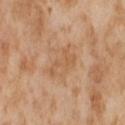follow-up=imaged on a skin check; not biopsied | patient=female, about 55 years old | body site=the right thigh | size=about 4.5 mm | lighting=cross-polarized illumination | image=~15 mm crop, total-body skin-cancer survey.